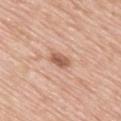workup: no biopsy performed (imaged during a skin exam) | subject: male, aged 78–82 | anatomic site: the mid back | image: 15 mm crop, total-body photography | tile lighting: white-light | TBP lesion metrics: an outline eccentricity of about 0.9 (0 = round, 1 = elongated) and a symmetry-axis asymmetry near 0.2; a border-irregularity index near 2.5/10, a color-variation rating of about 2/10, and a peripheral color-asymmetry measure near 1 | diameter: ≈3.5 mm.